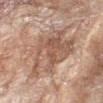The lesion was tiled from a total-body skin photograph and was not biopsied. This image is a 15 mm lesion crop taken from a total-body photograph. About 8 mm across. Automated tile analysis of the lesion measured a footprint of about 32 mm² and a shape-asymmetry score of about 0.3 (0 = symmetric). And it measured a border-irregularity rating of about 4.5/10, a within-lesion color-variation index near 6/10, and peripheral color asymmetry of about 2. And it measured a nevus-likeness score of about 0/100 and a detector confidence of about 95 out of 100 that the crop contains a lesion. This is a white-light tile. The lesion is on the left forearm. The patient is a female in their mid- to late 70s.